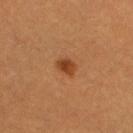Recorded during total-body skin imaging; not selected for excision or biopsy. The lesion's longest dimension is about 2.5 mm. Located on the left leg. A lesion tile, about 15 mm wide, cut from a 3D total-body photograph. A female subject about 30 years old. This is a cross-polarized tile.